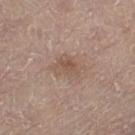Q: Was this lesion biopsied?
A: imaged on a skin check; not biopsied
Q: Automated lesion metrics?
A: an area of roughly 7 mm² and a symmetry-axis asymmetry near 0.3
Q: What is the imaging modality?
A: total-body-photography crop, ~15 mm field of view
Q: What are the patient's age and sex?
A: male, approximately 80 years of age
Q: How large is the lesion?
A: about 3.5 mm
Q: Illumination type?
A: white-light
Q: Where on the body is the lesion?
A: the left thigh